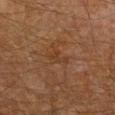Part of a total-body skin-imaging series; this lesion was reviewed on a skin check and was not flagged for biopsy. The subject is a male in their 50s. This image is a 15 mm lesion crop taken from a total-body photograph. Located on the arm. Captured under cross-polarized illumination. About 3 mm across.